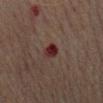{"biopsy_status": "not biopsied; imaged during a skin examination", "lighting": "cross-polarized", "image": {"source": "total-body photography crop", "field_of_view_mm": 15}, "site": "chest", "lesion_size": {"long_diameter_mm_approx": 2.5}, "patient": {"sex": "male", "age_approx": 70}}